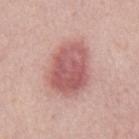Assessment:
Part of a total-body skin-imaging series; this lesion was reviewed on a skin check and was not flagged for biopsy.
Image and clinical context:
A male subject aged 53 to 57. From the mid back. The total-body-photography lesion software estimated a lesion area of about 23 mm², an outline eccentricity of about 0.7 (0 = round, 1 = elongated), and a symmetry-axis asymmetry near 0.15. The software also gave a lesion color around L≈57 a*≈26 b*≈23 in CIELAB, about 13 CIELAB-L* units darker than the surrounding skin, and a normalized lesion–skin contrast near 8.5. The software also gave a peripheral color-asymmetry measure near 1.5. The tile uses white-light illumination. A 15 mm close-up extracted from a 3D total-body photography capture.